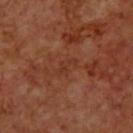The lesion was tiled from a total-body skin photograph and was not biopsied. A male subject aged 68 to 72. The lesion is on the back. Cropped from a whole-body photographic skin survey; the tile spans about 15 mm. Longest diameter approximately 3 mm. Automated image analysis of the tile measured a border-irregularity index near 6/10 and a color-variation rating of about 0/10. It also reported a nevus-likeness score of about 0/100 and a lesion-detection confidence of about 100/100.